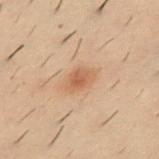Clinical impression: No biopsy was performed on this lesion — it was imaged during a full skin examination and was not determined to be concerning. Clinical summary: The recorded lesion diameter is about 3.5 mm. Automated tile analysis of the lesion measured a lesion color around L≈48 a*≈17 b*≈29 in CIELAB, roughly 8 lightness units darker than nearby skin, and a lesion-to-skin contrast of about 6 (normalized; higher = more distinct). The analysis additionally found a border-irregularity rating of about 2/10, internal color variation of about 3 on a 0–10 scale, and radial color variation of about 1. The analysis additionally found an automated nevus-likeness rating near 55 out of 100 and a lesion-detection confidence of about 100/100. The tile uses cross-polarized illumination. The lesion is located on the front of the torso. A 15 mm close-up tile from a total-body photography series done for melanoma screening. A male subject aged around 30.